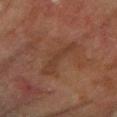Part of a total-body skin-imaging series; this lesion was reviewed on a skin check and was not flagged for biopsy.
The subject is a female about 80 years old.
The tile uses cross-polarized illumination.
The total-body-photography lesion software estimated an area of roughly 6.5 mm² and a shape-asymmetry score of about 0.55 (0 = symmetric). And it measured a mean CIELAB color near L≈32 a*≈18 b*≈25, roughly 5 lightness units darker than nearby skin, and a normalized border contrast of about 5. The software also gave a border-irregularity index near 7.5/10, a within-lesion color-variation index near 0.5/10, and peripheral color asymmetry of about 0.
A region of skin cropped from a whole-body photographic capture, roughly 15 mm wide.
The lesion is on the left forearm.
The recorded lesion diameter is about 6 mm.